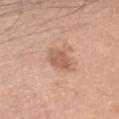biopsy_status: not biopsied; imaged during a skin examination
patient:
  sex: male
  age_approx: 50
site: head or neck
automated_metrics:
  eccentricity: 0.25
  shape_asymmetry: 0.15
  cielab_L: 59
  cielab_a: 21
  cielab_b: 31
  vs_skin_darker_L: 10.0
  vs_skin_contrast_norm: 6.5
  nevus_likeness_0_100: 15
image:
  source: total-body photography crop
  field_of_view_mm: 15